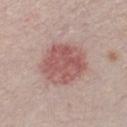workup=total-body-photography surveillance lesion; no biopsy
diameter=about 5.5 mm
patient=male, aged approximately 30
automated metrics=an area of roughly 21 mm², a shape eccentricity near 0.5, and a symmetry-axis asymmetry near 0.1; a nevus-likeness score of about 95/100 and lesion-presence confidence of about 100/100
acquisition=total-body-photography crop, ~15 mm field of view
illumination=white-light